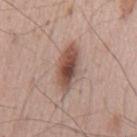workup = imaged on a skin check; not biopsied
patient = male, approximately 65 years of age
site = the chest
image = ~15 mm tile from a whole-body skin photo